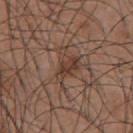Impression:
Recorded during total-body skin imaging; not selected for excision or biopsy.
Clinical summary:
An algorithmic analysis of the crop reported roughly 8 lightness units darker than nearby skin and a lesion-to-skin contrast of about 6.5 (normalized; higher = more distinct). Captured under white-light illumination. The lesion's longest dimension is about 5 mm. On the chest. The patient is a male in their mid- to late 50s. A close-up tile cropped from a whole-body skin photograph, about 15 mm across.